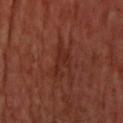Notes:
• workup: catalogued during a skin exam; not biopsied
• lesion size: ~5 mm (longest diameter)
• image source: total-body-photography crop, ~15 mm field of view
• patient: male, in their mid- to late 60s
• anatomic site: the head or neck
• image-analysis metrics: a footprint of about 8 mm² and an eccentricity of roughly 0.9; a mean CIELAB color near L≈28 a*≈25 b*≈26, a lesion–skin lightness drop of about 5, and a normalized lesion–skin contrast near 5.5; a border-irregularity index near 5.5/10 and a color-variation rating of about 2/10; a classifier nevus-likeness of about 0/100 and lesion-presence confidence of about 85/100
• lighting: cross-polarized